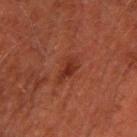No biopsy was performed on this lesion — it was imaged during a full skin examination and was not determined to be concerning. A close-up tile cropped from a whole-body skin photograph, about 15 mm across. A male patient, aged 43–47. The lesion is on the right upper arm. This is a cross-polarized tile. The total-body-photography lesion software estimated a border-irregularity rating of about 3.5/10, a within-lesion color-variation index near 2/10, and peripheral color asymmetry of about 0.5. The software also gave a classifier nevus-likeness of about 20/100.